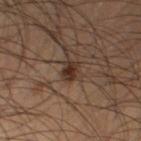biopsy status: catalogued during a skin exam; not biopsied | image source: ~15 mm tile from a whole-body skin photo | site: the right thigh | patient: male, in their mid- to late 60s.